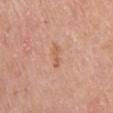| field | value |
|---|---|
| workup | catalogued during a skin exam; not biopsied |
| patient | male, aged around 60 |
| automated lesion analysis | a lesion-detection confidence of about 100/100 |
| image | ~15 mm tile from a whole-body skin photo |
| location | the upper back |